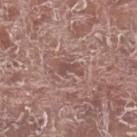Automated tile analysis of the lesion measured a lesion area of about 3 mm², an outline eccentricity of about 0.9 (0 = round, 1 = elongated), and two-axis asymmetry of about 0.4. The software also gave a nevus-likeness score of about 0/100 and a detector confidence of about 75 out of 100 that the crop contains a lesion. A male subject aged 73 to 77. A 15 mm close-up extracted from a 3D total-body photography capture. From the left lower leg.